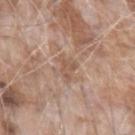Case summary:
• biopsy status · catalogued during a skin exam; not biopsied
• subject · male, in their mid- to late 70s
• image · ~15 mm tile from a whole-body skin photo
• TBP lesion metrics · a border-irregularity index near 5.5/10, a within-lesion color-variation index near 1.5/10, and peripheral color asymmetry of about 0.5; a classifier nevus-likeness of about 0/100 and lesion-presence confidence of about 95/100
• tile lighting · white-light
• site · the left forearm